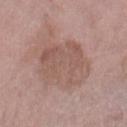| feature | finding |
|---|---|
| notes | catalogued during a skin exam; not biopsied |
| illumination | white-light |
| patient | female, approximately 70 years of age |
| lesion diameter | ≈7.5 mm |
| acquisition | total-body-photography crop, ~15 mm field of view |
| site | the right thigh |